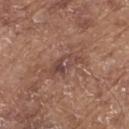Recorded during total-body skin imaging; not selected for excision or biopsy. The lesion is located on the upper back. A close-up tile cropped from a whole-body skin photograph, about 15 mm across. Measured at roughly 4 mm in maximum diameter. Automated tile analysis of the lesion measured a lesion area of about 5.5 mm² and an outline eccentricity of about 0.9 (0 = round, 1 = elongated). The analysis additionally found a lesion color around L≈45 a*≈20 b*≈23 in CIELAB and a normalized border contrast of about 7. It also reported border irregularity of about 5 on a 0–10 scale, internal color variation of about 6 on a 0–10 scale, and a peripheral color-asymmetry measure near 2. It also reported a nevus-likeness score of about 5/100. A male subject, aged around 80.